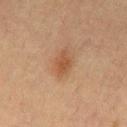{
  "biopsy_status": "not biopsied; imaged during a skin examination",
  "image": {
    "source": "total-body photography crop",
    "field_of_view_mm": 15
  },
  "site": "chest",
  "patient": {
    "sex": "male",
    "age_approx": 65
  }
}